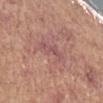Recorded during total-body skin imaging; not selected for excision or biopsy. A female subject, roughly 65 years of age. Cropped from a total-body skin-imaging series; the visible field is about 15 mm. From the left forearm. Imaged with white-light lighting. The recorded lesion diameter is about 4 mm. The total-body-photography lesion software estimated a normalized border contrast of about 5. The analysis additionally found a detector confidence of about 85 out of 100 that the crop contains a lesion.